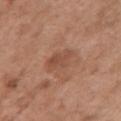Q: Automated lesion metrics?
A: a lesion area of about 6 mm², a shape eccentricity near 0.85, and a symmetry-axis asymmetry near 0.2; roughly 8 lightness units darker than nearby skin; an automated nevus-likeness rating near 0 out of 100
Q: What are the patient's age and sex?
A: female, aged 48–52
Q: What is the anatomic site?
A: the chest
Q: Illumination type?
A: white-light
Q: How was this image acquired?
A: ~15 mm tile from a whole-body skin photo
Q: What is the lesion's diameter?
A: ≈4 mm